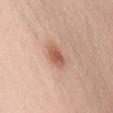follow-up: catalogued during a skin exam; not biopsied | image source: total-body-photography crop, ~15 mm field of view | anatomic site: the front of the torso | patient: female, aged 58 to 62.